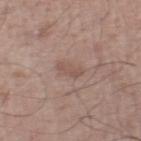Background:
A male subject, roughly 55 years of age. Automated image analysis of the tile measured border irregularity of about 3.5 on a 0–10 scale, a within-lesion color-variation index near 0.5/10, and a peripheral color-asymmetry measure near 0. A region of skin cropped from a whole-body photographic capture, roughly 15 mm wide. The lesion is on the leg. About 3 mm across. The tile uses white-light illumination.